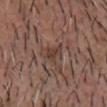A lesion tile, about 15 mm wide, cut from a 3D total-body photograph. About 2.5 mm across. A male subject, approximately 65 years of age. The lesion is on the chest. Automated tile analysis of the lesion measured border irregularity of about 4.5 on a 0–10 scale and peripheral color asymmetry of about 0.5. This is a white-light tile.